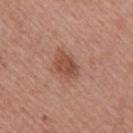  lesion_size:
    long_diameter_mm_approx: 3.5
  lighting: white-light
  automated_metrics:
    area_mm2_approx: 6.5
    eccentricity: 0.75
    shape_asymmetry: 0.25
  patient:
    sex: female
    age_approx: 55
  image:
    source: total-body photography crop
    field_of_view_mm: 15
  site: right upper arm